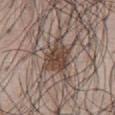Clinical impression:
The lesion was photographed on a routine skin check and not biopsied; there is no pathology result.